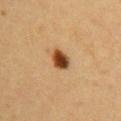The lesion was photographed on a routine skin check and not biopsied; there is no pathology result.
A female patient aged 28–32.
On the right upper arm.
A close-up tile cropped from a whole-body skin photograph, about 15 mm across.